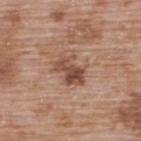• biopsy status · no biopsy performed (imaged during a skin exam)
• patient · male, in their 50s
• image source · 15 mm crop, total-body photography
• site · the upper back